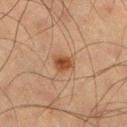Q: Was this lesion biopsied?
A: total-body-photography surveillance lesion; no biopsy
Q: What is the anatomic site?
A: the left thigh
Q: What is the imaging modality?
A: ~15 mm crop, total-body skin-cancer survey
Q: Automated lesion metrics?
A: a lesion area of about 4.5 mm² and a shape-asymmetry score of about 0.25 (0 = symmetric); border irregularity of about 2 on a 0–10 scale, a within-lesion color-variation index near 3/10, and a peripheral color-asymmetry measure near 1; a detector confidence of about 100 out of 100 that the crop contains a lesion
Q: How was the tile lit?
A: cross-polarized illumination
Q: What is the lesion's diameter?
A: ~2.5 mm (longest diameter)
Q: Patient demographics?
A: male, about 65 years old